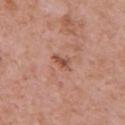Assessment:
Recorded during total-body skin imaging; not selected for excision or biopsy.
Clinical summary:
The lesion is on the upper back. The patient is a female aged 48–52. This image is a 15 mm lesion crop taken from a total-body photograph.